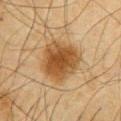workup: catalogued during a skin exam; not biopsied
image: total-body-photography crop, ~15 mm field of view
patient: male, aged 63 to 67
site: the chest